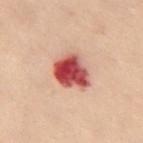The lesion was photographed on a routine skin check and not biopsied; there is no pathology result.
A 15 mm crop from a total-body photograph taken for skin-cancer surveillance.
The lesion-visualizer software estimated an average lesion color of about L≈44 a*≈32 b*≈25 (CIELAB), a lesion–skin lightness drop of about 19, and a normalized lesion–skin contrast near 13.5. It also reported internal color variation of about 8 on a 0–10 scale. It also reported a classifier nevus-likeness of about 0/100.
The subject is a female aged around 60.
About 4 mm across.
The lesion is located on the left leg.